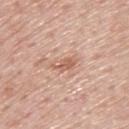notes=catalogued during a skin exam; not biopsied
subject=male, approximately 75 years of age
location=the upper back
lesion diameter=about 3 mm
lighting=white-light illumination
image=total-body-photography crop, ~15 mm field of view
image-analysis metrics=an area of roughly 3.5 mm²; a classifier nevus-likeness of about 15/100 and a lesion-detection confidence of about 100/100